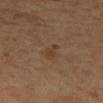Findings:
• notes: no biopsy performed (imaged during a skin exam)
• tile lighting: cross-polarized illumination
• lesion diameter: ~3 mm (longest diameter)
• automated lesion analysis: an automated nevus-likeness rating near 0 out of 100
• subject: female, in their 50s
• anatomic site: the right lower leg
• image: 15 mm crop, total-body photography